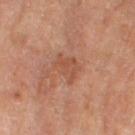Part of a total-body skin-imaging series; this lesion was reviewed on a skin check and was not flagged for biopsy. An algorithmic analysis of the crop reported a border-irregularity index near 4/10 and a color-variation rating of about 2/10. The software also gave a nevus-likeness score of about 0/100 and a detector confidence of about 100 out of 100 that the crop contains a lesion. Cropped from a whole-body photographic skin survey; the tile spans about 15 mm. Captured under cross-polarized illumination. The lesion is on the right thigh. A female subject aged around 70. About 4 mm across.